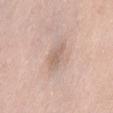follow-up: no biopsy performed (imaged during a skin exam)
lesion diameter: ~3.5 mm (longest diameter)
image source: total-body-photography crop, ~15 mm field of view
automated lesion analysis: a lesion area of about 5.5 mm², an eccentricity of roughly 0.8, and a symmetry-axis asymmetry near 0.25; a nevus-likeness score of about 0/100 and a detector confidence of about 100 out of 100 that the crop contains a lesion
patient: female, in their 60s
tile lighting: white-light illumination
location: the lower back